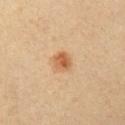biopsy status — imaged on a skin check; not biopsied
illumination — cross-polarized
image source — total-body-photography crop, ~15 mm field of view
location — the left upper arm
subject — female, in their 40s